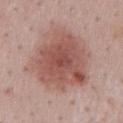| key | value |
|---|---|
| workup | no biopsy performed (imaged during a skin exam) |
| patient | male, aged approximately 50 |
| acquisition | ~15 mm crop, total-body skin-cancer survey |
| illumination | white-light illumination |
| size | ≈7.5 mm |
| body site | the mid back |